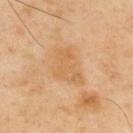Assessment:
The lesion was photographed on a routine skin check and not biopsied; there is no pathology result.
Clinical summary:
The lesion is located on the upper back. A 15 mm crop from a total-body photograph taken for skin-cancer surveillance. The lesion's longest dimension is about 5 mm. The patient is a male roughly 55 years of age. This is a cross-polarized tile.